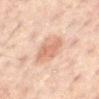workup: catalogued during a skin exam; not biopsied
lighting: cross-polarized
TBP lesion metrics: a border-irregularity index near 2/10, a within-lesion color-variation index near 2.5/10, and peripheral color asymmetry of about 1; a classifier nevus-likeness of about 70/100 and a detector confidence of about 100 out of 100 that the crop contains a lesion
acquisition: 15 mm crop, total-body photography
subject: male, in their 60s
body site: the mid back
lesion diameter: about 4 mm